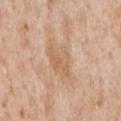Q: Was a biopsy performed?
A: catalogued during a skin exam; not biopsied
Q: Where on the body is the lesion?
A: the chest
Q: What kind of image is this?
A: ~15 mm tile from a whole-body skin photo
Q: What are the patient's age and sex?
A: male, aged approximately 65